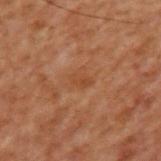Part of a total-body skin-imaging series; this lesion was reviewed on a skin check and was not flagged for biopsy. A male subject, about 70 years old. The lesion is located on the upper back. Longest diameter approximately 2.5 mm. Cropped from a total-body skin-imaging series; the visible field is about 15 mm. This is a cross-polarized tile.